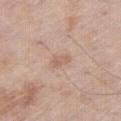workup: catalogued during a skin exam; not biopsied
patient: male, about 60 years old
size: ≈2.5 mm
body site: the right thigh
imaging modality: ~15 mm tile from a whole-body skin photo
automated lesion analysis: a lesion area of about 3.5 mm², a shape eccentricity near 0.85, and a shape-asymmetry score of about 0.25 (0 = symmetric); a mean CIELAB color near L≈61 a*≈19 b*≈28, about 7 CIELAB-L* units darker than the surrounding skin, and a normalized lesion–skin contrast near 5; border irregularity of about 2.5 on a 0–10 scale and a color-variation rating of about 2/10